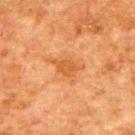notes = total-body-photography surveillance lesion; no biopsy
subject = male, about 80 years old
anatomic site = the upper back
image source = ~15 mm tile from a whole-body skin photo
image-analysis metrics = a footprint of about 5.5 mm²; roughly 7 lightness units darker than nearby skin and a normalized border contrast of about 6; a within-lesion color-variation index near 1.5/10 and a peripheral color-asymmetry measure near 0.5; an automated nevus-likeness rating near 0 out of 100 and lesion-presence confidence of about 100/100
size = ~4 mm (longest diameter)